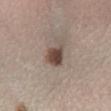Automated image analysis of the tile measured a lesion color around L≈45 a*≈14 b*≈22 in CIELAB and a normalized border contrast of about 10.5. The analysis additionally found a nevus-likeness score of about 95/100. A female subject aged 63–67. On the right lower leg. This image is a 15 mm lesion crop taken from a total-body photograph.